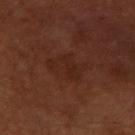This lesion was catalogued during total-body skin photography and was not selected for biopsy. The recorded lesion diameter is about 4 mm. An algorithmic analysis of the crop reported a nevus-likeness score of about 15/100. Cropped from a whole-body photographic skin survey; the tile spans about 15 mm. Located on the right upper arm. A male patient, aged around 60. The tile uses cross-polarized illumination.